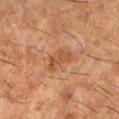| key | value |
|---|---|
| follow-up | no biopsy performed (imaged during a skin exam) |
| tile lighting | cross-polarized |
| diameter | about 3.5 mm |
| anatomic site | the left lower leg |
| subject | female, about 50 years old |
| image source | total-body-photography crop, ~15 mm field of view |